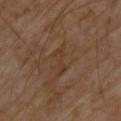{
  "biopsy_status": "not biopsied; imaged during a skin examination",
  "site": "upper back",
  "image": {
    "source": "total-body photography crop",
    "field_of_view_mm": 15
  },
  "lesion_size": {
    "long_diameter_mm_approx": 3.5
  },
  "lighting": "cross-polarized",
  "patient": {
    "sex": "male",
    "age_approx": 70
  }
}